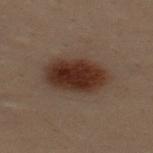This lesion was catalogued during total-body skin photography and was not selected for biopsy. A male patient aged approximately 30. A 15 mm close-up extracted from a 3D total-body photography capture. Captured under cross-polarized illumination. Automated tile analysis of the lesion measured a lesion area of about 19 mm² and two-axis asymmetry of about 0.15. The software also gave a lesion color around L≈24 a*≈15 b*≈20 in CIELAB, roughly 11 lightness units darker than nearby skin, and a lesion-to-skin contrast of about 12 (normalized; higher = more distinct). And it measured border irregularity of about 1.5 on a 0–10 scale and a within-lesion color-variation index near 4/10. From the back.